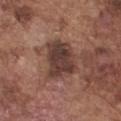workup = no biopsy performed (imaged during a skin exam)
site = the chest
subject = male, aged 73–77
illumination = white-light
image = ~15 mm tile from a whole-body skin photo